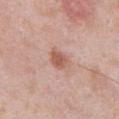Notes:
* patient — male, aged around 55
* lighting — white-light illumination
* size — ~3 mm (longest diameter)
* site — the abdomen
* acquisition — ~15 mm tile from a whole-body skin photo